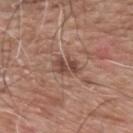Assessment:
Part of a total-body skin-imaging series; this lesion was reviewed on a skin check and was not flagged for biopsy.
Background:
About 3.5 mm across. The subject is a male aged 58–62. On the mid back. A close-up tile cropped from a whole-body skin photograph, about 15 mm across.